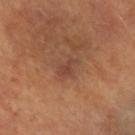Findings:
– biopsy status · catalogued during a skin exam; not biopsied
– automated lesion analysis · a lesion color around L≈44 a*≈23 b*≈28 in CIELAB; a peripheral color-asymmetry measure near 0.5; a detector confidence of about 100 out of 100 that the crop contains a lesion
– body site · the left forearm
– patient · female, aged 58–62
– illumination · cross-polarized
– image source · ~15 mm crop, total-body skin-cancer survey
– size · ≈3 mm